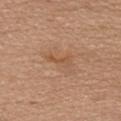| field | value |
|---|---|
| notes | total-body-photography surveillance lesion; no biopsy |
| site | the head or neck |
| image-analysis metrics | border irregularity of about 5.5 on a 0–10 scale, internal color variation of about 0 on a 0–10 scale, and radial color variation of about 0 |
| image source | 15 mm crop, total-body photography |
| patient | female, aged around 35 |
| tile lighting | white-light |
| lesion diameter | ~3.5 mm (longest diameter) |